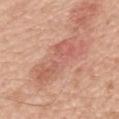Q: Is there a histopathology result?
A: no biopsy performed (imaged during a skin exam)
Q: How was this image acquired?
A: ~15 mm crop, total-body skin-cancer survey
Q: Where on the body is the lesion?
A: the mid back
Q: Patient demographics?
A: male, about 70 years old